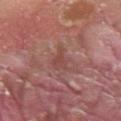Assessment: Part of a total-body skin-imaging series; this lesion was reviewed on a skin check and was not flagged for biopsy. Image and clinical context: This is a white-light tile. A 15 mm close-up extracted from a 3D total-body photography capture. From the left forearm. The lesion's longest dimension is about 3.5 mm. The lesion-visualizer software estimated a border-irregularity rating of about 4.5/10 and radial color variation of about 0.5. A male subject in their 40s.